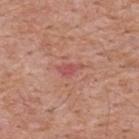Recorded during total-body skin imaging; not selected for excision or biopsy. The lesion is on the back. This image is a 15 mm lesion crop taken from a total-body photograph. A male patient, in their mid- to late 50s.